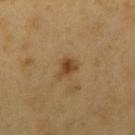Q: Was a biopsy performed?
A: no biopsy performed (imaged during a skin exam)
Q: Where on the body is the lesion?
A: the right upper arm
Q: Patient demographics?
A: male, approximately 60 years of age
Q: What kind of image is this?
A: ~15 mm crop, total-body skin-cancer survey
Q: Illumination type?
A: cross-polarized